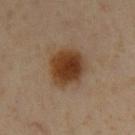Q: Is there a histopathology result?
A: no biopsy performed (imaged during a skin exam)
Q: What kind of image is this?
A: total-body-photography crop, ~15 mm field of view
Q: Illumination type?
A: cross-polarized illumination
Q: Where on the body is the lesion?
A: the chest
Q: What are the patient's age and sex?
A: male, aged 58 to 62
Q: Lesion size?
A: about 4.5 mm
Q: What did automated image analysis measure?
A: a lesion–skin lightness drop of about 12; a within-lesion color-variation index near 4.5/10; a nevus-likeness score of about 100/100 and a lesion-detection confidence of about 100/100